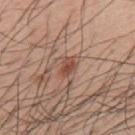Impression:
Part of a total-body skin-imaging series; this lesion was reviewed on a skin check and was not flagged for biopsy.
Image and clinical context:
The lesion is located on the upper back. A 15 mm close-up tile from a total-body photography series done for melanoma screening. The total-body-photography lesion software estimated a within-lesion color-variation index near 2.5/10 and a peripheral color-asymmetry measure near 1. The tile uses white-light illumination. The patient is a male aged 53 to 57. Approximately 2.5 mm at its widest.